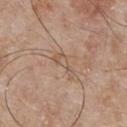<tbp_lesion>
  <biopsy_status>not biopsied; imaged during a skin examination</biopsy_status>
</tbp_lesion>